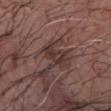Clinical impression: Recorded during total-body skin imaging; not selected for excision or biopsy. Acquisition and patient details: A male patient aged 68 to 72. A 15 mm close-up extracted from a 3D total-body photography capture. On the left forearm.